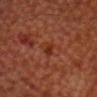anatomic site: the head or neck; image: ~15 mm tile from a whole-body skin photo; lighting: cross-polarized illumination; patient: male, in their mid- to late 60s.